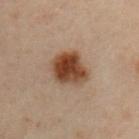Notes:
* biopsy status: catalogued during a skin exam; not biopsied
* lesion size: ~4.5 mm (longest diameter)
* lighting: cross-polarized
* image: 15 mm crop, total-body photography
* subject: male, aged 53 to 57
* location: the arm